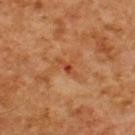Q: Was a biopsy performed?
A: catalogued during a skin exam; not biopsied
Q: What lighting was used for the tile?
A: cross-polarized illumination
Q: Lesion location?
A: the upper back
Q: What is the lesion's diameter?
A: ~3 mm (longest diameter)
Q: Who is the patient?
A: male, approximately 60 years of age
Q: What is the imaging modality?
A: total-body-photography crop, ~15 mm field of view